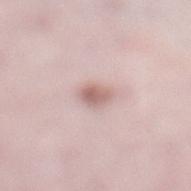Clinical impression:
No biopsy was performed on this lesion — it was imaged during a full skin examination and was not determined to be concerning.
Acquisition and patient details:
The recorded lesion diameter is about 2.5 mm. Automated tile analysis of the lesion measured about 11 CIELAB-L* units darker than the surrounding skin and a normalized border contrast of about 7. It also reported a nevus-likeness score of about 70/100. The lesion is located on the leg. Captured under white-light illumination. A female patient in their mid-40s. A 15 mm crop from a total-body photograph taken for skin-cancer surveillance.